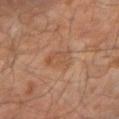This lesion was catalogued during total-body skin photography and was not selected for biopsy.